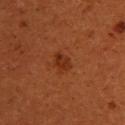notes = catalogued during a skin exam; not biopsied | subject = female, aged 28 to 32 | anatomic site = the upper back | lighting = cross-polarized | automated lesion analysis = a lesion color around L≈29 a*≈26 b*≈33 in CIELAB and roughly 7 lightness units darker than nearby skin; a nevus-likeness score of about 80/100 and a lesion-detection confidence of about 100/100 | image source = ~15 mm tile from a whole-body skin photo.